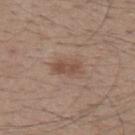| field | value |
|---|---|
| follow-up | catalogued during a skin exam; not biopsied |
| subject | male, about 40 years old |
| acquisition | ~15 mm crop, total-body skin-cancer survey |
| site | the upper back |